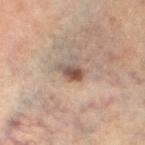Captured during whole-body skin photography for melanoma surveillance; the lesion was not biopsied.